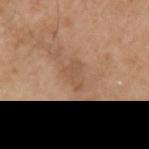Q: Was a biopsy performed?
A: imaged on a skin check; not biopsied
Q: What is the lesion's diameter?
A: about 3.5 mm
Q: What lighting was used for the tile?
A: white-light
Q: What are the patient's age and sex?
A: male, aged 53–57
Q: How was this image acquired?
A: ~15 mm tile from a whole-body skin photo
Q: Lesion location?
A: the left upper arm